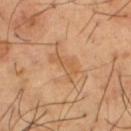Clinical impression: No biopsy was performed on this lesion — it was imaged during a full skin examination and was not determined to be concerning. Background: Automated image analysis of the tile measured a mean CIELAB color near L≈57 a*≈21 b*≈38, roughly 7 lightness units darker than nearby skin, and a normalized lesion–skin contrast near 5.5. The analysis additionally found an automated nevus-likeness rating near 0 out of 100 and a detector confidence of about 100 out of 100 that the crop contains a lesion. Captured under cross-polarized illumination. Located on the leg. The subject is a male approximately 65 years of age. Measured at roughly 4 mm in maximum diameter. A 15 mm crop from a total-body photograph taken for skin-cancer surveillance.